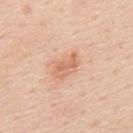<tbp_lesion>
  <biopsy_status>not biopsied; imaged during a skin examination</biopsy_status>
  <site>upper back</site>
  <image>
    <source>total-body photography crop</source>
    <field_of_view_mm>15</field_of_view_mm>
  </image>
  <patient>
    <sex>male</sex>
    <age_approx>50</age_approx>
  </patient>
</tbp_lesion>